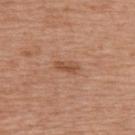No biopsy was performed on this lesion — it was imaged during a full skin examination and was not determined to be concerning.
The patient is a female aged 38–42.
This image is a 15 mm lesion crop taken from a total-body photograph.
Automated image analysis of the tile measured a mean CIELAB color near L≈52 a*≈23 b*≈32, a lesion–skin lightness drop of about 8, and a lesion-to-skin contrast of about 6.5 (normalized; higher = more distinct). And it measured a classifier nevus-likeness of about 15/100 and a detector confidence of about 100 out of 100 that the crop contains a lesion.
Imaged with white-light lighting.
The lesion's longest dimension is about 3 mm.
The lesion is on the back.